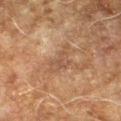{"biopsy_status": "not biopsied; imaged during a skin examination", "lighting": "cross-polarized", "site": "right forearm", "image": {"source": "total-body photography crop", "field_of_view_mm": 15}, "patient": {"sex": "male", "age_approx": 70}, "automated_metrics": {"eccentricity": 0.8, "shape_asymmetry": 0.55, "border_irregularity_0_10": 6.5, "color_variation_0_10": 1.5, "peripheral_color_asymmetry": 0.5, "nevus_likeness_0_100": 0, "lesion_detection_confidence_0_100": 70}}